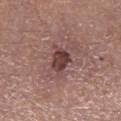| key | value |
|---|---|
| biopsy status | total-body-photography surveillance lesion; no biopsy |
| anatomic site | the right lower leg |
| subject | male, aged approximately 55 |
| lesion size | ≈5 mm |
| image-analysis metrics | a border-irregularity index near 5.5/10, a within-lesion color-variation index near 5/10, and radial color variation of about 1.5; an automated nevus-likeness rating near 5 out of 100 |
| illumination | white-light |
| image source | ~15 mm crop, total-body skin-cancer survey |